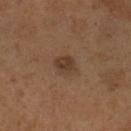notes: catalogued during a skin exam; not biopsied | diameter: about 2.5 mm | illumination: cross-polarized | subject: male, aged 53–57 | image: 15 mm crop, total-body photography | location: the leg.